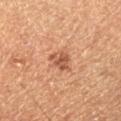– follow-up · imaged on a skin check; not biopsied
– acquisition · ~15 mm crop, total-body skin-cancer survey
– subject · male, aged around 65
– anatomic site · the right lower leg